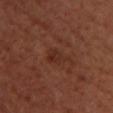The lesion was photographed on a routine skin check and not biopsied; there is no pathology result. This is a cross-polarized tile. The lesion's longest dimension is about 3.5 mm. The lesion is on the upper back. A roughly 15 mm field-of-view crop from a total-body skin photograph. A female subject, aged 38 to 42.